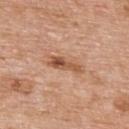{
  "lesion_size": {
    "long_diameter_mm_approx": 4.0
  },
  "patient": {
    "sex": "male",
    "age_approx": 60
  },
  "automated_metrics": {
    "vs_skin_darker_L": 12.0,
    "nevus_likeness_0_100": 70,
    "lesion_detection_confidence_0_100": 100
  },
  "site": "upper back",
  "image": {
    "source": "total-body photography crop",
    "field_of_view_mm": 15
  },
  "lighting": "white-light"
}